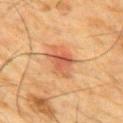Q: Was this lesion biopsied?
A: catalogued during a skin exam; not biopsied
Q: Lesion size?
A: ~4 mm (longest diameter)
Q: What is the imaging modality?
A: ~15 mm crop, total-body skin-cancer survey
Q: What lighting was used for the tile?
A: cross-polarized
Q: Patient demographics?
A: male, aged 83 or older
Q: What is the anatomic site?
A: the front of the torso